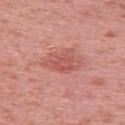Recorded during total-body skin imaging; not selected for excision or biopsy. The tile uses white-light illumination. The recorded lesion diameter is about 4.5 mm. The lesion is on the upper back. Automated tile analysis of the lesion measured a lesion area of about 8.5 mm², an eccentricity of roughly 0.85, and two-axis asymmetry of about 0.3. The software also gave a detector confidence of about 100 out of 100 that the crop contains a lesion. A region of skin cropped from a whole-body photographic capture, roughly 15 mm wide. The patient is a female about 40 years old.